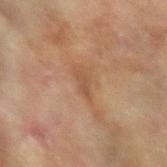The lesion was tiled from a total-body skin photograph and was not biopsied.
About 2.5 mm across.
The patient is a female about 80 years old.
A 15 mm crop from a total-body photograph taken for skin-cancer surveillance.
The lesion-visualizer software estimated a lesion area of about 2.5 mm² and two-axis asymmetry of about 0.35.
The lesion is located on the left forearm.